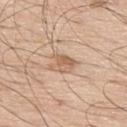Clinical impression:
The lesion was photographed on a routine skin check and not biopsied; there is no pathology result.
Background:
A roughly 15 mm field-of-view crop from a total-body skin photograph. Captured under white-light illumination. The lesion is on the upper back. The patient is a male approximately 70 years of age.